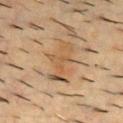The lesion was tiled from a total-body skin photograph and was not biopsied.
Approximately 5.5 mm at its widest.
Imaged with cross-polarized lighting.
A male patient about 55 years old.
A close-up tile cropped from a whole-body skin photograph, about 15 mm across.
Located on the upper back.